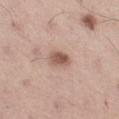Assessment: Recorded during total-body skin imaging; not selected for excision or biopsy. Context: Located on the right thigh. A male patient, roughly 55 years of age. Cropped from a total-body skin-imaging series; the visible field is about 15 mm.